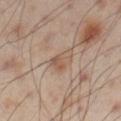Acquisition and patient details:
A male patient aged 53–57. This image is a 15 mm lesion crop taken from a total-body photograph. About 3 mm across. From the right thigh.